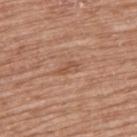follow-up = total-body-photography surveillance lesion; no biopsy
patient = female, about 60 years old
image = total-body-photography crop, ~15 mm field of view
site = the upper back